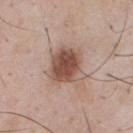Notes:
* biopsy status: total-body-photography surveillance lesion; no biopsy
* patient: male, approximately 55 years of age
* automated metrics: an outline eccentricity of about 0.3 (0 = round, 1 = elongated); an average lesion color of about L≈51 a*≈20 b*≈26 (CIELAB), roughly 14 lightness units darker than nearby skin, and a normalized lesion–skin contrast near 10; border irregularity of about 2.5 on a 0–10 scale and radial color variation of about 2
* site: the chest
* acquisition: 15 mm crop, total-body photography
* lesion diameter: ≈4.5 mm
* illumination: white-light illumination